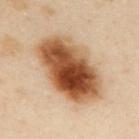A close-up tile cropped from a whole-body skin photograph, about 15 mm across. The subject is a female aged around 40. The lesion is located on the upper back. Measured at roughly 9 mm in maximum diameter. Automated tile analysis of the lesion measured a lesion area of about 38 mm², an outline eccentricity of about 0.8 (0 = round, 1 = elongated), and a symmetry-axis asymmetry near 0.15. It also reported a nevus-likeness score of about 100/100 and lesion-presence confidence of about 100/100. The tile uses cross-polarized illumination.